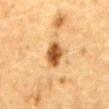biopsy status=imaged on a skin check; not biopsied
subject=male, aged 83 or over
location=the abdomen
image=~15 mm tile from a whole-body skin photo